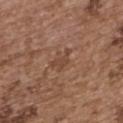Q: Was this lesion biopsied?
A: imaged on a skin check; not biopsied
Q: How was the tile lit?
A: white-light
Q: What is the anatomic site?
A: the back
Q: How large is the lesion?
A: ≈3 mm
Q: What kind of image is this?
A: ~15 mm tile from a whole-body skin photo
Q: Who is the patient?
A: female, aged around 65